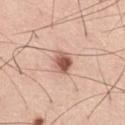Imaged during a routine full-body skin examination; the lesion was not biopsied and no histopathology is available. Located on the right thigh. Approximately 2.5 mm at its widest. A male subject in their mid-50s. A region of skin cropped from a whole-body photographic capture, roughly 15 mm wide. An algorithmic analysis of the crop reported a lesion area of about 5 mm² and a shape-asymmetry score of about 0.25 (0 = symmetric). And it measured border irregularity of about 2 on a 0–10 scale, a within-lesion color-variation index near 6/10, and a peripheral color-asymmetry measure near 2.5. The software also gave a classifier nevus-likeness of about 95/100 and lesion-presence confidence of about 100/100.